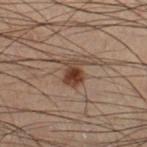• follow-up: catalogued during a skin exam; not biopsied
• subject: male, aged 53–57
• tile lighting: cross-polarized
• image: ~15 mm crop, total-body skin-cancer survey
• site: the right lower leg